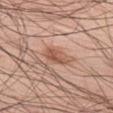On the leg. The lesion-visualizer software estimated an area of roughly 5 mm², a shape eccentricity near 0.8, and a shape-asymmetry score of about 0.2 (0 = symmetric). And it measured a mean CIELAB color near L≈55 a*≈23 b*≈30 and a lesion–skin lightness drop of about 10. And it measured a border-irregularity rating of about 2/10, a within-lesion color-variation index near 4/10, and peripheral color asymmetry of about 1.5. The analysis additionally found a lesion-detection confidence of about 100/100. A 15 mm crop from a total-body photograph taken for skin-cancer surveillance. A male patient, in their 60s.